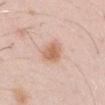- workup — catalogued during a skin exam; not biopsied
- lesion diameter — about 3 mm
- illumination — white-light
- image-analysis metrics — a lesion area of about 7 mm², an eccentricity of roughly 0.65, and a shape-asymmetry score of about 0.2 (0 = symmetric); an average lesion color of about L≈64 a*≈21 b*≈31 (CIELAB), about 10 CIELAB-L* units darker than the surrounding skin, and a normalized lesion–skin contrast near 7.5; a classifier nevus-likeness of about 95/100
- patient — male, aged 28–32
- acquisition — total-body-photography crop, ~15 mm field of view
- site — the left upper arm